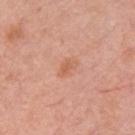Q: Is there a histopathology result?
A: no biopsy performed (imaged during a skin exam)
Q: What are the patient's age and sex?
A: female, aged 63 to 67
Q: What kind of image is this?
A: ~15 mm tile from a whole-body skin photo
Q: Lesion size?
A: ~2.5 mm (longest diameter)
Q: What did automated image analysis measure?
A: a mean CIELAB color near L≈61 a*≈26 b*≈34, a lesion–skin lightness drop of about 7, and a normalized lesion–skin contrast near 5.5; border irregularity of about 3 on a 0–10 scale and radial color variation of about 1
Q: What is the anatomic site?
A: the right upper arm
Q: How was the tile lit?
A: white-light